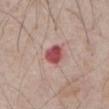biopsy status: catalogued during a skin exam; not biopsied
image: ~15 mm crop, total-body skin-cancer survey
automated lesion analysis: an area of roughly 5.5 mm² and an eccentricity of roughly 0.1; a lesion color around L≈50 a*≈30 b*≈21 in CIELAB, about 15 CIELAB-L* units darker than the surrounding skin, and a lesion-to-skin contrast of about 10.5 (normalized; higher = more distinct); a border-irregularity index near 2/10, internal color variation of about 4 on a 0–10 scale, and a peripheral color-asymmetry measure near 1.5; a nevus-likeness score of about 0/100 and lesion-presence confidence of about 100/100
subject: male, about 65 years old
anatomic site: the front of the torso